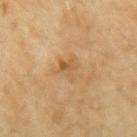notes = total-body-photography surveillance lesion; no biopsy | patient = female, roughly 55 years of age | image source = ~15 mm crop, total-body skin-cancer survey | site = the right forearm.